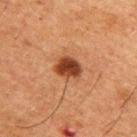notes: catalogued during a skin exam; not biopsied | subject: male, aged 48–52 | image-analysis metrics: a lesion area of about 7 mm² and an outline eccentricity of about 0.6 (0 = round, 1 = elongated); an automated nevus-likeness rating near 100 out of 100 and a lesion-detection confidence of about 100/100 | lesion size: about 3.5 mm | site: the back | imaging modality: ~15 mm tile from a whole-body skin photo.